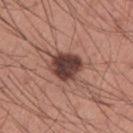{"biopsy_status": "not biopsied; imaged during a skin examination", "image": {"source": "total-body photography crop", "field_of_view_mm": 15}, "site": "left upper arm", "patient": {"sex": "male", "age_approx": 35}}